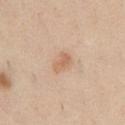Part of a total-body skin-imaging series; this lesion was reviewed on a skin check and was not flagged for biopsy.
The tile uses white-light illumination.
Cropped from a total-body skin-imaging series; the visible field is about 15 mm.
The lesion's longest dimension is about 2.5 mm.
A male subject aged around 35.
On the chest.